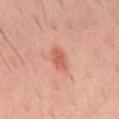This lesion was catalogued during total-body skin photography and was not selected for biopsy.
This is a cross-polarized tile.
Located on the mid back.
Longest diameter approximately 2.5 mm.
A male patient, in their 40s.
This image is a 15 mm lesion crop taken from a total-body photograph.